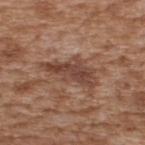No biopsy was performed on this lesion — it was imaged during a full skin examination and was not determined to be concerning. Located on the back. Approximately 6.5 mm at its widest. A lesion tile, about 15 mm wide, cut from a 3D total-body photograph. Automated image analysis of the tile measured an area of roughly 12 mm², a shape eccentricity near 0.9, and a shape-asymmetry score of about 0.4 (0 = symmetric). And it measured an average lesion color of about L≈44 a*≈20 b*≈27 (CIELAB), a lesion–skin lightness drop of about 10, and a normalized lesion–skin contrast near 8. It also reported a border-irregularity rating of about 6/10, a within-lesion color-variation index near 4.5/10, and radial color variation of about 1.5. A male subject, aged 63 to 67.